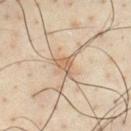Q: Was this lesion biopsied?
A: no biopsy performed (imaged during a skin exam)
Q: Patient demographics?
A: male, in their mid-40s
Q: Automated lesion metrics?
A: an area of roughly 5.5 mm², an outline eccentricity of about 0.5 (0 = round, 1 = elongated), and a symmetry-axis asymmetry near 0.45; a mean CIELAB color near L≈62 a*≈16 b*≈31, about 9 CIELAB-L* units darker than the surrounding skin, and a normalized lesion–skin contrast near 6; a border-irregularity index near 5.5/10, internal color variation of about 4 on a 0–10 scale, and peripheral color asymmetry of about 1.5; an automated nevus-likeness rating near 10 out of 100
Q: Where on the body is the lesion?
A: the chest
Q: How was this image acquired?
A: total-body-photography crop, ~15 mm field of view
Q: Illumination type?
A: cross-polarized
Q: What is the lesion's diameter?
A: ≈3 mm